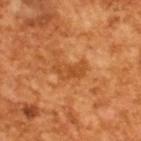This lesion was catalogued during total-body skin photography and was not selected for biopsy. A 15 mm close-up extracted from a 3D total-body photography capture. Automated tile analysis of the lesion measured an area of roughly 5 mm², an outline eccentricity of about 0.9 (0 = round, 1 = elongated), and two-axis asymmetry of about 0.35. And it measured a border-irregularity rating of about 4/10, a color-variation rating of about 3/10, and a peripheral color-asymmetry measure near 1. The analysis additionally found an automated nevus-likeness rating near 0 out of 100 and a detector confidence of about 100 out of 100 that the crop contains a lesion. The tile uses cross-polarized illumination. The lesion's longest dimension is about 3.5 mm. The patient is a male in their mid-60s.